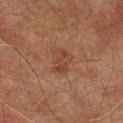follow-up: imaged on a skin check; not biopsied | patient: male, aged around 75 | lesion diameter: ≈3.5 mm | body site: the left thigh | image source: 15 mm crop, total-body photography | illumination: cross-polarized illumination.